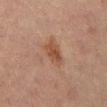biopsy status=imaged on a skin check; not biopsied
automated metrics=a lesion color around L≈39 a*≈18 b*≈26 in CIELAB and about 7 CIELAB-L* units darker than the surrounding skin; a nevus-likeness score of about 80/100 and lesion-presence confidence of about 100/100
patient=male, aged 63–67
anatomic site=the mid back
lesion size=~3.5 mm (longest diameter)
tile lighting=cross-polarized illumination
imaging modality=~15 mm crop, total-body skin-cancer survey